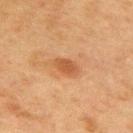Captured during whole-body skin photography for melanoma surveillance; the lesion was not biopsied. Measured at roughly 3 mm in maximum diameter. A close-up tile cropped from a whole-body skin photograph, about 15 mm across. The lesion is located on the mid back. A male patient in their mid-70s. This is a cross-polarized tile.